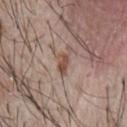This lesion was catalogued during total-body skin photography and was not selected for biopsy.
A region of skin cropped from a whole-body photographic capture, roughly 15 mm wide.
Imaged with white-light lighting.
Automated image analysis of the tile measured a classifier nevus-likeness of about 65/100.
The lesion is located on the chest.
A male subject in their mid-60s.
About 2.5 mm across.